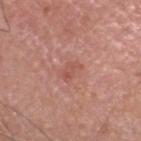Captured during whole-body skin photography for melanoma surveillance; the lesion was not biopsied.
A roughly 15 mm field-of-view crop from a total-body skin photograph.
The lesion is on the head or neck.
Automated image analysis of the tile measured an area of roughly 3.5 mm². The analysis additionally found a nevus-likeness score of about 0/100.
The subject is a male roughly 65 years of age.
The tile uses white-light illumination.